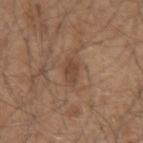<lesion>
<biopsy_status>not biopsied; imaged during a skin examination</biopsy_status>
<patient>
  <sex>male</sex>
  <age_approx>50</age_approx>
</patient>
<image>
  <source>total-body photography crop</source>
  <field_of_view_mm>15</field_of_view_mm>
</image>
<automated_metrics>
  <cielab_L>44</cielab_L>
  <cielab_a>18</cielab_a>
  <cielab_b>29</cielab_b>
  <vs_skin_darker_L>8.0</vs_skin_darker_L>
  <vs_skin_contrast_norm>6.0</vs_skin_contrast_norm>
  <nevus_likeness_0_100>15</nevus_likeness_0_100>
  <lesion_detection_confidence_0_100>100</lesion_detection_confidence_0_100>
</automated_metrics>
<lesion_size>
  <long_diameter_mm_approx>3.0</long_diameter_mm_approx>
</lesion_size>
<lighting>white-light</lighting>
<site>arm</site>
</lesion>